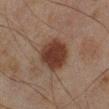Q: Was a biopsy performed?
A: catalogued during a skin exam; not biopsied
Q: How was the tile lit?
A: cross-polarized illumination
Q: What is the anatomic site?
A: the right lower leg
Q: What kind of image is this?
A: 15 mm crop, total-body photography
Q: What did automated image analysis measure?
A: border irregularity of about 1.5 on a 0–10 scale, a color-variation rating of about 3/10, and a peripheral color-asymmetry measure near 1
Q: Patient demographics?
A: male, aged around 45
Q: How large is the lesion?
A: ≈4.5 mm